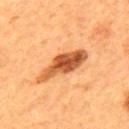Notes:
* imaging modality — 15 mm crop, total-body photography
* location — the mid back
* TBP lesion metrics — a lesion color around L≈52 a*≈28 b*≈42 in CIELAB, about 17 CIELAB-L* units darker than the surrounding skin, and a normalized border contrast of about 11; a border-irregularity index near 4/10, internal color variation of about 6.5 on a 0–10 scale, and peripheral color asymmetry of about 2.5
* subject — male, aged 53–57
* lesion size — about 6.5 mm